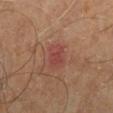Imaged during a routine full-body skin examination; the lesion was not biopsied and no histopathology is available.
This is a cross-polarized tile.
The total-body-photography lesion software estimated an average lesion color of about L≈42 a*≈25 b*≈25 (CIELAB), a lesion–skin lightness drop of about 6, and a normalized lesion–skin contrast near 6. The software also gave a border-irregularity rating of about 2/10 and a color-variation rating of about 3/10. It also reported an automated nevus-likeness rating near 0 out of 100 and lesion-presence confidence of about 100/100.
This image is a 15 mm lesion crop taken from a total-body photograph.
From the back.
The subject is a male roughly 60 years of age.